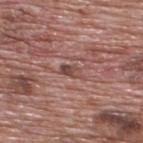Recorded during total-body skin imaging; not selected for excision or biopsy. A region of skin cropped from a whole-body photographic capture, roughly 15 mm wide. A male subject aged 68 to 72. This is a white-light tile. Located on the upper back. About 3 mm across.